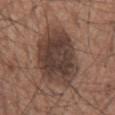Assessment:
The lesion was photographed on a routine skin check and not biopsied; there is no pathology result.
Background:
An algorithmic analysis of the crop reported a lesion area of about 33 mm², a shape eccentricity near 0.8, and a symmetry-axis asymmetry near 0.15. And it measured a border-irregularity rating of about 2/10, internal color variation of about 4.5 on a 0–10 scale, and peripheral color asymmetry of about 1.5. And it measured a nevus-likeness score of about 45/100. A lesion tile, about 15 mm wide, cut from a 3D total-body photograph. The lesion's longest dimension is about 9 mm. A male subject, approximately 75 years of age. The lesion is located on the mid back.